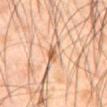The lesion was tiled from a total-body skin photograph and was not biopsied. Captured under cross-polarized illumination. About 3.5 mm across. Automated tile analysis of the lesion measured an area of roughly 4 mm² and an eccentricity of roughly 0.85. The software also gave a lesion color around L≈61 a*≈20 b*≈35 in CIELAB and about 11 CIELAB-L* units darker than the surrounding skin. A male patient aged around 65. A lesion tile, about 15 mm wide, cut from a 3D total-body photograph. The lesion is on the front of the torso.